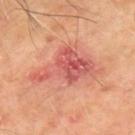Impression:
Imaged during a routine full-body skin examination; the lesion was not biopsied and no histopathology is available.
Image and clinical context:
A male subject, aged around 65. Imaged with cross-polarized lighting. The lesion is located on the arm. Automated tile analysis of the lesion measured a footprint of about 20 mm², a shape eccentricity near 0.85, and two-axis asymmetry of about 0.5. The analysis additionally found an average lesion color of about L≈56 a*≈31 b*≈29 (CIELAB), roughly 10 lightness units darker than nearby skin, and a normalized border contrast of about 7.5. It also reported a border-irregularity index near 7/10 and a within-lesion color-variation index near 5.5/10. And it measured a classifier nevus-likeness of about 0/100 and a lesion-detection confidence of about 100/100. The lesion's longest dimension is about 8.5 mm. Cropped from a total-body skin-imaging series; the visible field is about 15 mm.